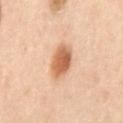lighting: cross-polarized illumination | diameter: about 4.5 mm | automated metrics: a lesion color around L≈62 a*≈24 b*≈37 in CIELAB, about 15 CIELAB-L* units darker than the surrounding skin, and a normalized border contrast of about 9.5; a border-irregularity index near 2/10 and a color-variation rating of about 4/10 | site: the mid back | image: total-body-photography crop, ~15 mm field of view | patient: male, aged 63 to 67.